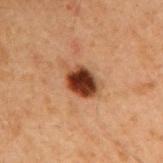Q: Was a biopsy performed?
A: no biopsy performed (imaged during a skin exam)
Q: Where on the body is the lesion?
A: the right upper arm
Q: Patient demographics?
A: male, aged 58 to 62
Q: How was this image acquired?
A: ~15 mm crop, total-body skin-cancer survey